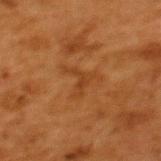| key | value |
|---|---|
| follow-up | imaged on a skin check; not biopsied |
| location | the back |
| subject | female, aged approximately 50 |
| tile lighting | cross-polarized |
| TBP lesion metrics | a mean CIELAB color near L≈34 a*≈22 b*≈34, about 5 CIELAB-L* units darker than the surrounding skin, and a lesion-to-skin contrast of about 5 (normalized; higher = more distinct) |
| lesion size | ~4.5 mm (longest diameter) |
| image source | ~15 mm tile from a whole-body skin photo |